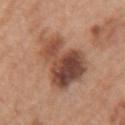follow-up — total-body-photography surveillance lesion; no biopsy | tile lighting — white-light | subject — female, aged approximately 60 | diameter — ≈8.5 mm | location — the right forearm | automated lesion analysis — an area of roughly 28 mm² and two-axis asymmetry of about 0.4; a lesion color around L≈48 a*≈21 b*≈29 in CIELAB, about 14 CIELAB-L* units darker than the surrounding skin, and a normalized lesion–skin contrast near 10; a border-irregularity index near 5/10, a within-lesion color-variation index near 10/10, and a peripheral color-asymmetry measure near 4 | image source — ~15 mm tile from a whole-body skin photo.